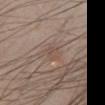A 15 mm close-up extracted from a 3D total-body photography capture. A male patient, approximately 40 years of age. Automated tile analysis of the lesion measured an area of roughly 5 mm², an eccentricity of roughly 0.65, and two-axis asymmetry of about 0.35. And it measured a border-irregularity index near 3.5/10 and peripheral color asymmetry of about 1. It also reported an automated nevus-likeness rating near 0 out of 100 and a lesion-detection confidence of about 75/100. The tile uses white-light illumination. The lesion is on the chest.